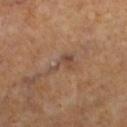Notes:
– notes · imaged on a skin check; not biopsied
– acquisition · ~15 mm crop, total-body skin-cancer survey
– automated metrics · a lesion area of about 4.5 mm² and an eccentricity of roughly 0.85; an average lesion color of about L≈43 a*≈17 b*≈26 (CIELAB) and roughly 7 lightness units darker than nearby skin; a classifier nevus-likeness of about 0/100
– body site · the left lower leg
– lesion diameter · ≈3.5 mm
– tile lighting · cross-polarized
– subject · female, in their mid-60s